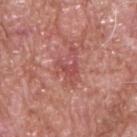The tile uses white-light illumination.
A male subject in their 60s.
A lesion tile, about 15 mm wide, cut from a 3D total-body photograph.
The total-body-photography lesion software estimated an area of roughly 2.5 mm², an eccentricity of roughly 0.85, and two-axis asymmetry of about 0.55. And it measured an average lesion color of about L≈49 a*≈31 b*≈25 (CIELAB), roughly 8 lightness units darker than nearby skin, and a normalized lesion–skin contrast near 5.5. The analysis additionally found an automated nevus-likeness rating near 0 out of 100 and a lesion-detection confidence of about 90/100.
The recorded lesion diameter is about 2.5 mm.
The lesion is on the upper back.